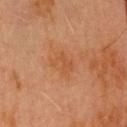Imaged during a routine full-body skin examination; the lesion was not biopsied and no histopathology is available.
The tile uses cross-polarized illumination.
The patient is a male aged around 70.
From the head or neck.
The total-body-photography lesion software estimated a lesion area of about 6 mm² and a shape-asymmetry score of about 0.25 (0 = symmetric). It also reported a color-variation rating of about 2/10 and peripheral color asymmetry of about 1. The analysis additionally found a classifier nevus-likeness of about 0/100 and lesion-presence confidence of about 100/100.
Cropped from a whole-body photographic skin survey; the tile spans about 15 mm.
The recorded lesion diameter is about 3 mm.